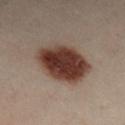The lesion was photographed on a routine skin check and not biopsied; there is no pathology result. A lesion tile, about 15 mm wide, cut from a 3D total-body photograph. The patient is a male aged approximately 50. Located on the left leg.